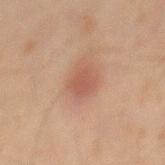Q: Was a biopsy performed?
A: no biopsy performed (imaged during a skin exam)
Q: What kind of image is this?
A: total-body-photography crop, ~15 mm field of view
Q: How large is the lesion?
A: about 3 mm
Q: Who is the patient?
A: male, approximately 45 years of age
Q: What is the anatomic site?
A: the mid back